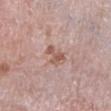Assessment: No biopsy was performed on this lesion — it was imaged during a full skin examination and was not determined to be concerning. Background: The patient is a male aged 73–77. This is a white-light tile. Cropped from a whole-body photographic skin survey; the tile spans about 15 mm. The lesion is on the left lower leg. An algorithmic analysis of the crop reported a border-irregularity index near 5/10, a within-lesion color-variation index near 1.5/10, and peripheral color asymmetry of about 0.5. It also reported an automated nevus-likeness rating near 0 out of 100 and lesion-presence confidence of about 100/100.